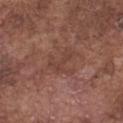| feature | finding |
|---|---|
| follow-up | total-body-photography surveillance lesion; no biopsy |
| subject | male, aged around 75 |
| automated lesion analysis | a mean CIELAB color near L≈41 a*≈21 b*≈25, about 5 CIELAB-L* units darker than the surrounding skin, and a lesion-to-skin contrast of about 4.5 (normalized; higher = more distinct) |
| acquisition | ~15 mm crop, total-body skin-cancer survey |
| location | the chest |